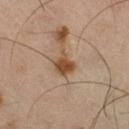The lesion is located on the right thigh. Imaged with cross-polarized lighting. The recorded lesion diameter is about 3.5 mm. A region of skin cropped from a whole-body photographic capture, roughly 15 mm wide. The patient is a male in their mid-40s.